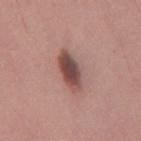Case summary:
* follow-up — imaged on a skin check; not biopsied
* imaging modality — 15 mm crop, total-body photography
* subject — male, aged around 35
* body site — the back